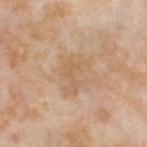Recorded during total-body skin imaging; not selected for excision or biopsy. The lesion-visualizer software estimated an area of roughly 8 mm², an eccentricity of roughly 0.8, and a symmetry-axis asymmetry near 0.45. The analysis additionally found an average lesion color of about L≈61 a*≈17 b*≈35 (CIELAB) and a lesion–skin lightness drop of about 6. Located on the left thigh. A 15 mm close-up tile from a total-body photography series done for melanoma screening. About 4.5 mm across. The tile uses cross-polarized illumination. A female subject roughly 55 years of age.